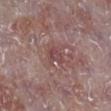Imaged during a routine full-body skin examination; the lesion was not biopsied and no histopathology is available. Approximately 2.5 mm at its widest. A male subject, aged approximately 75. A 15 mm close-up extracted from a 3D total-body photography capture. The lesion is located on the right lower leg. Automated tile analysis of the lesion measured a lesion area of about 3 mm² and a symmetry-axis asymmetry near 0.35. And it measured a border-irregularity index near 3/10 and peripheral color asymmetry of about 0.5.